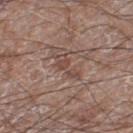follow-up: imaged on a skin check; not biopsied | lesion diameter: ~4.5 mm (longest diameter) | illumination: white-light illumination | image-analysis metrics: an eccentricity of roughly 0.95 and a shape-asymmetry score of about 0.45 (0 = symmetric); a lesion color around L≈46 a*≈18 b*≈23 in CIELAB, roughly 8 lightness units darker than nearby skin, and a normalized border contrast of about 6; a within-lesion color-variation index near 1/10 | imaging modality: ~15 mm tile from a whole-body skin photo | subject: male, approximately 60 years of age | site: the right lower leg.